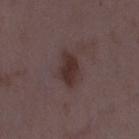biopsy status = no biopsy performed (imaged during a skin exam) | illumination = white-light | location = the right thigh | image = 15 mm crop, total-body photography | size = ≈4 mm | subject = female, aged around 35.